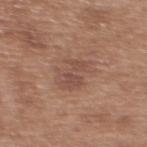Clinical impression:
Imaged during a routine full-body skin examination; the lesion was not biopsied and no histopathology is available.
Context:
A 15 mm close-up extracted from a 3D total-body photography capture. On the upper back. The tile uses white-light illumination. About 4.5 mm across. A female subject, roughly 40 years of age.